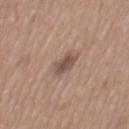Captured during whole-body skin photography for melanoma surveillance; the lesion was not biopsied. Imaged with white-light lighting. The lesion is located on the mid back. Measured at roughly 3 mm in maximum diameter. A male subject in their mid- to late 60s. A 15 mm crop from a total-body photograph taken for skin-cancer surveillance. Automated image analysis of the tile measured a lesion color around L≈51 a*≈17 b*≈23 in CIELAB, about 10 CIELAB-L* units darker than the surrounding skin, and a lesion-to-skin contrast of about 7.5 (normalized; higher = more distinct). The software also gave a border-irregularity rating of about 2.5/10, a within-lesion color-variation index near 2.5/10, and peripheral color asymmetry of about 1. And it measured lesion-presence confidence of about 100/100.